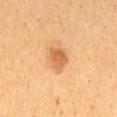Background:
The patient is a male roughly 45 years of age. This is a cross-polarized tile. A roughly 15 mm field-of-view crop from a total-body skin photograph. An algorithmic analysis of the crop reported a footprint of about 6 mm², a shape eccentricity near 0.55, and two-axis asymmetry of about 0.25. The software also gave internal color variation of about 2.5 on a 0–10 scale and radial color variation of about 1. It also reported an automated nevus-likeness rating near 90 out of 100 and a detector confidence of about 100 out of 100 that the crop contains a lesion. Located on the front of the torso. The recorded lesion diameter is about 3 mm.